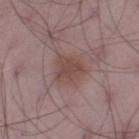| key | value |
|---|---|
| follow-up | imaged on a skin check; not biopsied |
| patient | male, aged approximately 50 |
| location | the left thigh |
| image | ~15 mm crop, total-body skin-cancer survey |
| lesion diameter | about 3.5 mm |
| tile lighting | white-light |